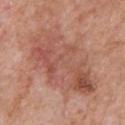Recorded during total-body skin imaging; not selected for excision or biopsy.
From the chest.
This is a white-light tile.
Longest diameter approximately 11 mm.
Cropped from a whole-body photographic skin survey; the tile spans about 15 mm.
An algorithmic analysis of the crop reported an eccentricity of roughly 0.8 and two-axis asymmetry of about 0.2. And it measured border irregularity of about 4 on a 0–10 scale, internal color variation of about 7 on a 0–10 scale, and radial color variation of about 2.5. The analysis additionally found a nevus-likeness score of about 0/100.
A male subject aged 58 to 62.